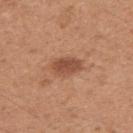Imaged during a routine full-body skin examination; the lesion was not biopsied and no histopathology is available. The total-body-photography lesion software estimated a lesion color around L≈50 a*≈24 b*≈32 in CIELAB, about 11 CIELAB-L* units darker than the surrounding skin, and a normalized lesion–skin contrast near 7.5. The analysis additionally found a lesion-detection confidence of about 100/100. From the right upper arm. Captured under white-light illumination. A female patient, aged approximately 30. The recorded lesion diameter is about 3.5 mm. Cropped from a total-body skin-imaging series; the visible field is about 15 mm.